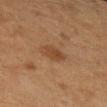Q: Was this lesion biopsied?
A: total-body-photography surveillance lesion; no biopsy
Q: What is the imaging modality?
A: ~15 mm tile from a whole-body skin photo
Q: Illumination type?
A: cross-polarized
Q: Automated lesion metrics?
A: roughly 7 lightness units darker than nearby skin and a normalized border contrast of about 6.5
Q: Patient demographics?
A: female, roughly 40 years of age
Q: Where on the body is the lesion?
A: the left upper arm
Q: How large is the lesion?
A: ≈2.5 mm